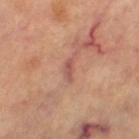notes — imaged on a skin check; not biopsied | tile lighting — cross-polarized | TBP lesion metrics — a footprint of about 3.5 mm², a shape eccentricity near 0.9, and a symmetry-axis asymmetry near 0.55; an automated nevus-likeness rating near 0 out of 100 and a lesion-detection confidence of about 80/100 | patient — female, aged approximately 60 | location — the right leg | image source — ~15 mm crop, total-body skin-cancer survey.